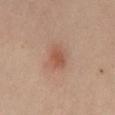The lesion was photographed on a routine skin check and not biopsied; there is no pathology result. About 3 mm across. The total-body-photography lesion software estimated a mean CIELAB color near L≈50 a*≈22 b*≈29 and a lesion-to-skin contrast of about 6.5 (normalized; higher = more distinct). The analysis additionally found a border-irregularity index near 2.5/10, internal color variation of about 2 on a 0–10 scale, and radial color variation of about 1. It also reported a nevus-likeness score of about 90/100 and lesion-presence confidence of about 100/100. On the mid back. This is a cross-polarized tile. The subject is a male in their mid-30s. A 15 mm crop from a total-body photograph taken for skin-cancer surveillance.